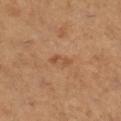notes = total-body-photography surveillance lesion; no biopsy
TBP lesion metrics = border irregularity of about 4.5 on a 0–10 scale, a within-lesion color-variation index near 0/10, and a peripheral color-asymmetry measure near 0; a classifier nevus-likeness of about 0/100 and lesion-presence confidence of about 100/100
subject = female, roughly 55 years of age
image source = 15 mm crop, total-body photography
size = ~3 mm (longest diameter)
anatomic site = the right lower leg